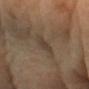Imaged during a routine full-body skin examination; the lesion was not biopsied and no histopathology is available. The subject is a female approximately 70 years of age. Located on the left forearm. A close-up tile cropped from a whole-body skin photograph, about 15 mm across. The recorded lesion diameter is about 2.5 mm.